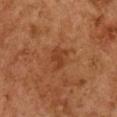Impression:
Imaged during a routine full-body skin examination; the lesion was not biopsied and no histopathology is available.
Context:
The recorded lesion diameter is about 3 mm. A female patient in their 60s. An algorithmic analysis of the crop reported a mean CIELAB color near L≈35 a*≈23 b*≈32, a lesion–skin lightness drop of about 6, and a normalized lesion–skin contrast near 6. The lesion is located on the chest. A 15 mm close-up tile from a total-body photography series done for melanoma screening.